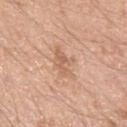{"biopsy_status": "not biopsied; imaged during a skin examination", "lighting": "white-light", "site": "left upper arm", "image": {"source": "total-body photography crop", "field_of_view_mm": 15}, "lesion_size": {"long_diameter_mm_approx": 3.5}, "patient": {"sex": "male", "age_approx": 20}}